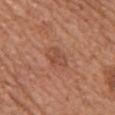The lesion was tiled from a total-body skin photograph and was not biopsied.
Cropped from a total-body skin-imaging series; the visible field is about 15 mm.
The patient is a female in their mid-50s.
On the chest.